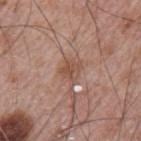Captured during whole-body skin photography for melanoma surveillance; the lesion was not biopsied.
Approximately 2.5 mm at its widest.
Located on the left upper arm.
A male patient aged 63 to 67.
A close-up tile cropped from a whole-body skin photograph, about 15 mm across.
An algorithmic analysis of the crop reported an area of roughly 4.5 mm² and a symmetry-axis asymmetry near 0.2. It also reported a border-irregularity rating of about 2/10, a color-variation rating of about 3/10, and a peripheral color-asymmetry measure near 1.
Imaged with white-light lighting.